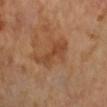The lesion was tiled from a total-body skin photograph and was not biopsied. The lesion's longest dimension is about 5.5 mm. The lesion-visualizer software estimated two-axis asymmetry of about 0.45. The analysis additionally found border irregularity of about 6 on a 0–10 scale, a within-lesion color-variation index near 2/10, and radial color variation of about 0.5. And it measured a lesion-detection confidence of about 100/100. A 15 mm close-up tile from a total-body photography series done for melanoma screening. The tile uses cross-polarized illumination. A female patient aged around 65. Located on the left arm.